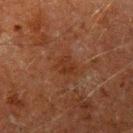Impression:
Captured during whole-body skin photography for melanoma surveillance; the lesion was not biopsied.
Clinical summary:
A 15 mm close-up extracted from a 3D total-body photography capture. The patient is a male aged 58–62. The lesion is located on the left upper arm. Automated image analysis of the tile measured an average lesion color of about L≈26 a*≈19 b*≈26 (CIELAB), roughly 5 lightness units darker than nearby skin, and a normalized lesion–skin contrast near 6. The software also gave a detector confidence of about 100 out of 100 that the crop contains a lesion. Measured at roughly 2.5 mm in maximum diameter.